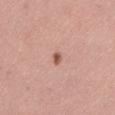Assessment: Imaged during a routine full-body skin examination; the lesion was not biopsied and no histopathology is available. Background: From the leg. A close-up tile cropped from a whole-body skin photograph, about 15 mm across. A female patient, approximately 35 years of age.